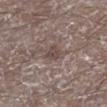biopsy status: imaged on a skin check; not biopsied
site: the left lower leg
tile lighting: white-light illumination
size: ≈2.5 mm
image: ~15 mm tile from a whole-body skin photo
subject: male, about 65 years old